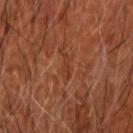<record>
  <biopsy_status>not biopsied; imaged during a skin examination</biopsy_status>
  <lighting>cross-polarized</lighting>
  <lesion_size>
    <long_diameter_mm_approx>3.0</long_diameter_mm_approx>
  </lesion_size>
  <automated_metrics>
    <area_mm2_approx>2.0</area_mm2_approx>
    <eccentricity>0.95</eccentricity>
    <shape_asymmetry>0.35</shape_asymmetry>
    <cielab_L>36</cielab_L>
    <cielab_a>25</cielab_a>
    <cielab_b>30</cielab_b>
    <vs_skin_darker_L>6.0</vs_skin_darker_L>
    <vs_skin_contrast_norm>5.0</vs_skin_contrast_norm>
  </automated_metrics>
  <patient>
    <sex>male</sex>
    <age_approx>65</age_approx>
  </patient>
  <image>
    <source>total-body photography crop</source>
    <field_of_view_mm>15</field_of_view_mm>
  </image>
</record>